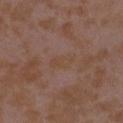<tbp_lesion>
  <biopsy_status>not biopsied; imaged during a skin examination</biopsy_status>
  <patient>
    <sex>female</sex>
    <age_approx>35</age_approx>
  </patient>
  <site>arm</site>
  <lighting>white-light</lighting>
  <lesion_size>
    <long_diameter_mm_approx>3.0</long_diameter_mm_approx>
  </lesion_size>
  <image>
    <source>total-body photography crop</source>
    <field_of_view_mm>15</field_of_view_mm>
  </image>
</tbp_lesion>